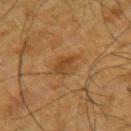- workup: total-body-photography surveillance lesion; no biopsy
- anatomic site: the arm
- lesion diameter: ~3.5 mm (longest diameter)
- subject: male, about 65 years old
- image source: 15 mm crop, total-body photography
- lighting: cross-polarized illumination